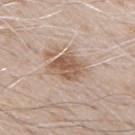Q: Was this lesion biopsied?
A: catalogued during a skin exam; not biopsied
Q: What are the patient's age and sex?
A: male, about 70 years old
Q: Lesion location?
A: the chest
Q: What is the imaging modality?
A: ~15 mm crop, total-body skin-cancer survey
Q: What lighting was used for the tile?
A: white-light
Q: What is the lesion's diameter?
A: about 4 mm
Q: Automated lesion metrics?
A: a lesion color around L≈57 a*≈16 b*≈29 in CIELAB, about 12 CIELAB-L* units darker than the surrounding skin, and a normalized border contrast of about 8